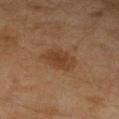  image:
    source: total-body photography crop
    field_of_view_mm: 15
  site: right upper arm
  automated_metrics:
    area_mm2_approx: 8.0
    eccentricity: 0.8
  patient:
    sex: female
    age_approx: 60
  lighting: cross-polarized
  lesion_size:
    long_diameter_mm_approx: 4.5